Automated image analysis of the tile measured an area of roughly 10 mm², a shape eccentricity near 0.8, and two-axis asymmetry of about 0.2. It also reported a lesion–skin lightness drop of about 15 and a normalized border contrast of about 10.5. The software also gave lesion-presence confidence of about 100/100 · measured at roughly 4.5 mm in maximum diameter · located on the upper back · a close-up tile cropped from a whole-body skin photograph, about 15 mm across · a male patient about 55 years old · captured under white-light illumination:
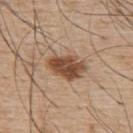Case summary:
– diagnosis · a dysplastic (Clark) nevus — a benign lesion